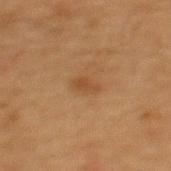Recorded during total-body skin imaging; not selected for excision or biopsy.
A male subject, aged approximately 65.
From the mid back.
The total-body-photography lesion software estimated a lesion color around L≈39 a*≈17 b*≈32 in CIELAB, a lesion–skin lightness drop of about 6, and a normalized border contrast of about 5.5.
A region of skin cropped from a whole-body photographic capture, roughly 15 mm wide.